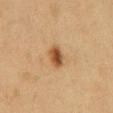  image:
    source: total-body photography crop
    field_of_view_mm: 15
  lesion_size:
    long_diameter_mm_approx: 2.5
  site: chest
  lighting: cross-polarized
  patient:
    sex: male
    age_approx: 55